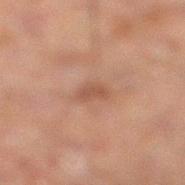The lesion was photographed on a routine skin check and not biopsied; there is no pathology result.
Located on the leg.
Imaged with cross-polarized lighting.
A male patient, about 60 years old.
The lesion-visualizer software estimated a lesion area of about 4 mm², an outline eccentricity of about 0.75 (0 = round, 1 = elongated), and a symmetry-axis asymmetry near 0.25. The analysis additionally found a lesion color around L≈39 a*≈17 b*≈24 in CIELAB. It also reported a border-irregularity index near 2.5/10 and radial color variation of about 0.5. The analysis additionally found an automated nevus-likeness rating near 0 out of 100 and a detector confidence of about 100 out of 100 that the crop contains a lesion.
Cropped from a whole-body photographic skin survey; the tile spans about 15 mm.
The lesion's longest dimension is about 2.5 mm.